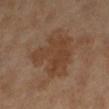Impression:
No biopsy was performed on this lesion — it was imaged during a full skin examination and was not determined to be concerning.
Clinical summary:
The lesion-visualizer software estimated an average lesion color of about L≈41 a*≈18 b*≈30 (CIELAB) and about 7 CIELAB-L* units darker than the surrounding skin. The analysis additionally found a border-irregularity index near 5/10, a within-lesion color-variation index near 3/10, and a peripheral color-asymmetry measure near 1. The software also gave a classifier nevus-likeness of about 0/100 and a lesion-detection confidence of about 100/100. A lesion tile, about 15 mm wide, cut from a 3D total-body photograph. Measured at roughly 6 mm in maximum diameter. The subject is a female aged around 55. The lesion is located on the left lower leg.